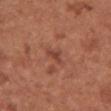<record>
<site>chest</site>
<automated_metrics>
  <color_variation_0_10>0.0</color_variation_0_10>
  <peripheral_color_asymmetry>0.0</peripheral_color_asymmetry>
  <nevus_likeness_0_100>0</nevus_likeness_0_100>
</automated_metrics>
<patient>
  <sex>female</sex>
  <age_approx>50</age_approx>
</patient>
<lighting>white-light</lighting>
<image>
  <source>total-body photography crop</source>
  <field_of_view_mm>15</field_of_view_mm>
</image>
</record>